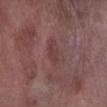Part of a total-body skin-imaging series; this lesion was reviewed on a skin check and was not flagged for biopsy.
The subject is a male aged around 75.
The total-body-photography lesion software estimated a nevus-likeness score of about 0/100.
A lesion tile, about 15 mm wide, cut from a 3D total-body photograph.
From the left lower leg.